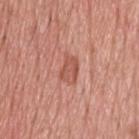biopsy status — no biopsy performed (imaged during a skin exam); subject — male, approximately 70 years of age; site — the head or neck; size — ~3 mm (longest diameter); illumination — white-light illumination; image — 15 mm crop, total-body photography.